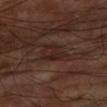The lesion was tiled from a total-body skin photograph and was not biopsied. Captured under cross-polarized illumination. Automated image analysis of the tile measured a footprint of about 7.5 mm², an eccentricity of roughly 0.6, and a shape-asymmetry score of about 0.3 (0 = symmetric). The analysis additionally found a border-irregularity rating of about 3.5/10, a within-lesion color-variation index near 2.5/10, and radial color variation of about 1. It also reported a detector confidence of about 75 out of 100 that the crop contains a lesion. A 15 mm crop from a total-body photograph taken for skin-cancer surveillance. Measured at roughly 3.5 mm in maximum diameter. A male patient, aged approximately 60. From the arm.